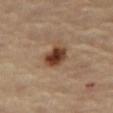{"biopsy_status": "not biopsied; imaged during a skin examination", "image": {"source": "total-body photography crop", "field_of_view_mm": 15}, "site": "abdomen", "patient": {"sex": "female", "age_approx": 70}}